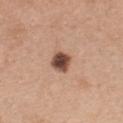Notes:
• notes: no biopsy performed (imaged during a skin exam)
• image source: 15 mm crop, total-body photography
• body site: the chest
• subject: female, aged 28 to 32
• diameter: ≈2.5 mm